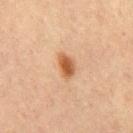Q: Was a biopsy performed?
A: catalogued during a skin exam; not biopsied
Q: Illumination type?
A: cross-polarized illumination
Q: What is the imaging modality?
A: ~15 mm tile from a whole-body skin photo
Q: Patient demographics?
A: male, aged 63 to 67
Q: Automated lesion metrics?
A: an eccentricity of roughly 0.8; a lesion–skin lightness drop of about 11 and a lesion-to-skin contrast of about 9.5 (normalized; higher = more distinct); border irregularity of about 2.5 on a 0–10 scale, a color-variation rating of about 3/10, and radial color variation of about 1
Q: Lesion size?
A: about 3 mm
Q: What is the anatomic site?
A: the upper back